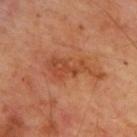follow-up = catalogued during a skin exam; not biopsied
tile lighting = cross-polarized illumination
site = the upper back
subject = male, aged around 65
image source = total-body-photography crop, ~15 mm field of view
size = about 6.5 mm